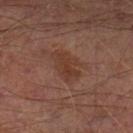The lesion was tiled from a total-body skin photograph and was not biopsied.